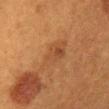An algorithmic analysis of the crop reported border irregularity of about 3.5 on a 0–10 scale, internal color variation of about 4 on a 0–10 scale, and radial color variation of about 1.5. And it measured a nevus-likeness score of about 0/100 and lesion-presence confidence of about 100/100. Approximately 5.5 mm at its widest. On the front of the torso. The patient is a female roughly 40 years of age. A close-up tile cropped from a whole-body skin photograph, about 15 mm across.